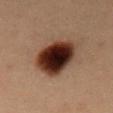The lesion was tiled from a total-body skin photograph and was not biopsied. A roughly 15 mm field-of-view crop from a total-body skin photograph. Measured at roughly 6 mm in maximum diameter. The lesion is located on the mid back. A male patient approximately 55 years of age.